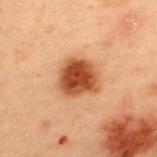This lesion was catalogued during total-body skin photography and was not selected for biopsy.
Cropped from a whole-body photographic skin survey; the tile spans about 15 mm.
The lesion is located on the upper back.
The subject is a male aged 53–57.